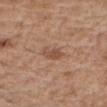Q: What is the imaging modality?
A: 15 mm crop, total-body photography
Q: Lesion location?
A: the right forearm
Q: Patient demographics?
A: male, roughly 70 years of age
Q: What is the lesion's diameter?
A: ≈3 mm